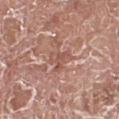Part of a total-body skin-imaging series; this lesion was reviewed on a skin check and was not flagged for biopsy. Captured under white-light illumination. A male subject, aged approximately 75. Cropped from a total-body skin-imaging series; the visible field is about 15 mm. Measured at roughly 3 mm in maximum diameter. On the left lower leg.